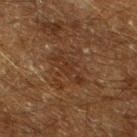- follow-up: imaged on a skin check; not biopsied